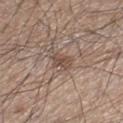Assessment:
Imaged during a routine full-body skin examination; the lesion was not biopsied and no histopathology is available.
Background:
On the right lower leg. An algorithmic analysis of the crop reported a lesion color around L≈48 a*≈16 b*≈24 in CIELAB, roughly 9 lightness units darker than nearby skin, and a normalized lesion–skin contrast near 7. It also reported a border-irregularity index near 3/10, a color-variation rating of about 2/10, and a peripheral color-asymmetry measure near 0.5. It also reported an automated nevus-likeness rating near 40 out of 100 and a lesion-detection confidence of about 90/100. Imaged with white-light lighting. A male subject aged around 45. The lesion's longest dimension is about 2.5 mm. Cropped from a whole-body photographic skin survey; the tile spans about 15 mm.